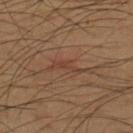Assessment:
No biopsy was performed on this lesion — it was imaged during a full skin examination and was not determined to be concerning.
Acquisition and patient details:
A roughly 15 mm field-of-view crop from a total-body skin photograph. The recorded lesion diameter is about 4.5 mm. Located on the upper back. An algorithmic analysis of the crop reported a lesion–skin lightness drop of about 6 and a lesion-to-skin contrast of about 5.5 (normalized; higher = more distinct). The software also gave an automated nevus-likeness rating near 0 out of 100 and a lesion-detection confidence of about 85/100. The tile uses cross-polarized illumination. The patient is a male aged 48 to 52.